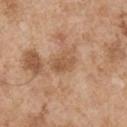Clinical impression: This lesion was catalogued during total-body skin photography and was not selected for biopsy. Background: Cropped from a whole-body photographic skin survey; the tile spans about 15 mm. Captured under white-light illumination. An algorithmic analysis of the crop reported an area of roughly 4.5 mm², an eccentricity of roughly 0.8, and two-axis asymmetry of about 0.2. The analysis additionally found roughly 9 lightness units darker than nearby skin and a normalized border contrast of about 6.5. And it measured border irregularity of about 2 on a 0–10 scale and a peripheral color-asymmetry measure near 1. A male patient, in their mid-50s. The lesion is on the right upper arm. Approximately 3 mm at its widest.